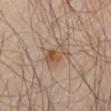biopsy status=no biopsy performed (imaged during a skin exam) | size=~3.5 mm (longest diameter) | TBP lesion metrics=an area of roughly 6.5 mm², a shape eccentricity near 0.75, and a symmetry-axis asymmetry near 0.35; an automated nevus-likeness rating near 75 out of 100 and lesion-presence confidence of about 100/100 | subject=male, approximately 65 years of age | illumination=cross-polarized | image=15 mm crop, total-body photography | location=the left thigh.